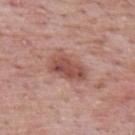Clinical impression: The lesion was photographed on a routine skin check and not biopsied; there is no pathology result.